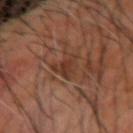| feature | finding |
|---|---|
| biopsy status | no biopsy performed (imaged during a skin exam) |
| patient | male, approximately 65 years of age |
| TBP lesion metrics | an average lesion color of about L≈36 a*≈21 b*≈28 (CIELAB) and a lesion–skin lightness drop of about 7; border irregularity of about 4.5 on a 0–10 scale and internal color variation of about 3 on a 0–10 scale |
| lesion size | about 3.5 mm |
| image | ~15 mm crop, total-body skin-cancer survey |
| illumination | cross-polarized |
| anatomic site | the arm |